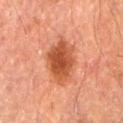Assessment:
Part of a total-body skin-imaging series; this lesion was reviewed on a skin check and was not flagged for biopsy.
Acquisition and patient details:
Captured under cross-polarized illumination. About 6 mm across. A male patient, aged 58 to 62. On the mid back. A roughly 15 mm field-of-view crop from a total-body skin photograph.